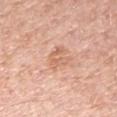Q: Is there a histopathology result?
A: total-body-photography surveillance lesion; no biopsy
Q: Patient demographics?
A: female, approximately 65 years of age
Q: Lesion location?
A: the arm
Q: How was this image acquired?
A: ~15 mm tile from a whole-body skin photo
Q: Lesion size?
A: about 3 mm